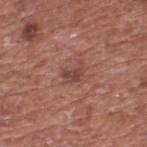Q: Was a biopsy performed?
A: imaged on a skin check; not biopsied
Q: Where on the body is the lesion?
A: the upper back
Q: Automated lesion metrics?
A: a border-irregularity index near 5/10, a within-lesion color-variation index near 2/10, and peripheral color asymmetry of about 1
Q: How was this image acquired?
A: ~15 mm tile from a whole-body skin photo
Q: Patient demographics?
A: male, aged 73–77
Q: Lesion size?
A: ≈2.5 mm
Q: How was the tile lit?
A: white-light illumination